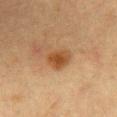Notes:
• biopsy status — imaged on a skin check; not biopsied
• subject — female, aged around 55
• location — the front of the torso
• image source — total-body-photography crop, ~15 mm field of view
• illumination — cross-polarized illumination